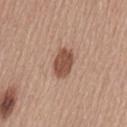notes = catalogued during a skin exam; not biopsied | body site = the lower back | acquisition = total-body-photography crop, ~15 mm field of view | lesion size = ≈3.5 mm | subject = female, aged 53–57.